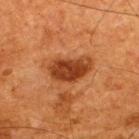The lesion was tiled from a total-body skin photograph and was not biopsied. Automated image analysis of the tile measured a footprint of about 11 mm², an eccentricity of roughly 0.85, and two-axis asymmetry of about 0.25. It also reported border irregularity of about 2.5 on a 0–10 scale, a within-lesion color-variation index near 3.5/10, and a peripheral color-asymmetry measure near 1. The analysis additionally found a detector confidence of about 100 out of 100 that the crop contains a lesion. A male patient about 65 years old. A 15 mm close-up tile from a total-body photography series done for melanoma screening. The recorded lesion diameter is about 5 mm. Imaged with cross-polarized lighting. The lesion is located on the back.